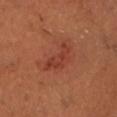Case summary:
– notes — no biopsy performed (imaged during a skin exam)
– imaging modality — total-body-photography crop, ~15 mm field of view
– location — the chest
– subject — male, aged 53 to 57